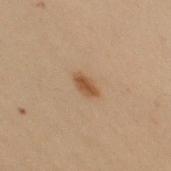This lesion was catalogued during total-body skin photography and was not selected for biopsy. Cropped from a whole-body photographic skin survey; the tile spans about 15 mm. Captured under cross-polarized illumination. Automated tile analysis of the lesion measured a lesion color around L≈44 a*≈17 b*≈30 in CIELAB. The analysis additionally found a classifier nevus-likeness of about 100/100. The lesion is on the upper back. The recorded lesion diameter is about 2.5 mm. A female patient, approximately 50 years of age.